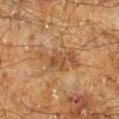Assessment:
Recorded during total-body skin imaging; not selected for excision or biopsy.
Image and clinical context:
The subject is a male aged 58 to 62. About 5.5 mm across. Cropped from a whole-body photographic skin survey; the tile spans about 15 mm. Located on the right lower leg.